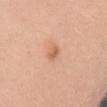| field | value |
|---|---|
| workup | imaged on a skin check; not biopsied |
| lesion diameter | ~2.5 mm (longest diameter) |
| subject | female, in their 30s |
| image source | total-body-photography crop, ~15 mm field of view |
| lighting | white-light illumination |
| body site | the arm |